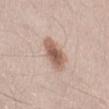| feature | finding |
|---|---|
| follow-up | no biopsy performed (imaged during a skin exam) |
| subject | male, in their mid-50s |
| tile lighting | white-light illumination |
| TBP lesion metrics | an outline eccentricity of about 0.75 (0 = round, 1 = elongated) and two-axis asymmetry of about 0.2; an average lesion color of about L≈59 a*≈18 b*≈27 (CIELAB) |
| lesion diameter | ≈4 mm |
| acquisition | 15 mm crop, total-body photography |
| anatomic site | the lower back |